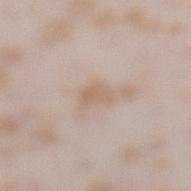This lesion was catalogued during total-body skin photography and was not selected for biopsy. A female subject aged around 25. This is a white-light tile. This image is a 15 mm lesion crop taken from a total-body photograph. From the left lower leg.